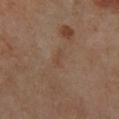The lesion was tiled from a total-body skin photograph and was not biopsied.
Imaged with cross-polarized lighting.
Measured at roughly 2.5 mm in maximum diameter.
Located on the right lower leg.
A female patient in their mid- to late 50s.
A lesion tile, about 15 mm wide, cut from a 3D total-body photograph.